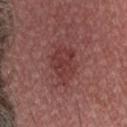Assessment: The lesion was tiled from a total-body skin photograph and was not biopsied. Acquisition and patient details: This is a white-light tile. A 15 mm close-up extracted from a 3D total-body photography capture. A male patient roughly 30 years of age. The lesion's longest dimension is about 4.5 mm.